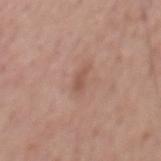biopsy_status: not biopsied; imaged during a skin examination
patient:
  sex: male
  age_approx: 70
lesion_size:
  long_diameter_mm_approx: 2.5
image:
  source: total-body photography crop
  field_of_view_mm: 15
site: back
lighting: white-light